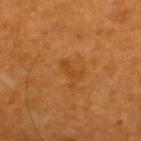Assessment: The lesion was photographed on a routine skin check and not biopsied; there is no pathology result. Context: An algorithmic analysis of the crop reported an area of roughly 2.5 mm² and two-axis asymmetry of about 0.45. The software also gave a lesion color around L≈42 a*≈25 b*≈41 in CIELAB and a lesion-to-skin contrast of about 5 (normalized; higher = more distinct). The analysis additionally found a border-irregularity rating of about 4.5/10. On the upper back. A male patient aged around 60. About 3 mm across. A roughly 15 mm field-of-view crop from a total-body skin photograph.